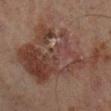Automated image analysis of the tile measured a lesion area of about 55 mm² and a symmetry-axis asymmetry near 0.5. And it measured a normalized lesion–skin contrast near 7. It also reported a border-irregularity index near 8/10, a within-lesion color-variation index near 5.5/10, and a peripheral color-asymmetry measure near 1.5. The lesion is located on the left lower leg. The tile uses cross-polarized illumination. The recorded lesion diameter is about 13 mm. A 15 mm close-up extracted from a 3D total-body photography capture. A male subject approximately 65 years of age.